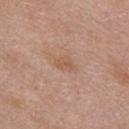Q: Is there a histopathology result?
A: catalogued during a skin exam; not biopsied
Q: What is the imaging modality?
A: 15 mm crop, total-body photography
Q: What is the lesion's diameter?
A: about 2.5 mm
Q: What is the anatomic site?
A: the front of the torso
Q: Who is the patient?
A: female, about 40 years old
Q: How was the tile lit?
A: white-light illumination